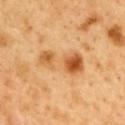Impression: Imaged during a routine full-body skin examination; the lesion was not biopsied and no histopathology is available. Context: The lesion's longest dimension is about 6.5 mm. A male patient about 50 years old. Cropped from a total-body skin-imaging series; the visible field is about 15 mm. Imaged with cross-polarized lighting. From the back.